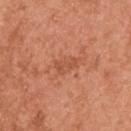biopsy_status: not biopsied; imaged during a skin examination
site: upper back
patient:
  sex: male
  age_approx: 55
image:
  source: total-body photography crop
  field_of_view_mm: 15